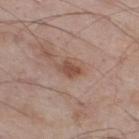The lesion was photographed on a routine skin check and not biopsied; there is no pathology result. The tile uses white-light illumination. A male patient, aged 53–57. Located on the right thigh. Automated tile analysis of the lesion measured a footprint of about 4.5 mm². The analysis additionally found a lesion color around L≈50 a*≈20 b*≈27 in CIELAB and a lesion-to-skin contrast of about 7.5 (normalized; higher = more distinct). It also reported a lesion-detection confidence of about 100/100. A roughly 15 mm field-of-view crop from a total-body skin photograph. Approximately 3 mm at its widest.